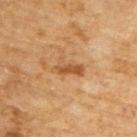No biopsy was performed on this lesion — it was imaged during a full skin examination and was not determined to be concerning. The total-body-photography lesion software estimated an area of roughly 5 mm² and a symmetry-axis asymmetry near 0.35. It also reported a mean CIELAB color near L≈48 a*≈20 b*≈37, about 9 CIELAB-L* units darker than the surrounding skin, and a normalized border contrast of about 7. The analysis additionally found a border-irregularity index near 5/10, a within-lesion color-variation index near 2/10, and radial color variation of about 0.5. And it measured a classifier nevus-likeness of about 15/100 and a detector confidence of about 100 out of 100 that the crop contains a lesion. A roughly 15 mm field-of-view crop from a total-body skin photograph. A male patient roughly 65 years of age. Measured at roughly 3.5 mm in maximum diameter. Located on the upper back.